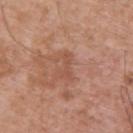biopsy_status: not biopsied; imaged during a skin examination
image:
  source: total-body photography crop
  field_of_view_mm: 15
site: upper back
lighting: white-light
lesion_size:
  long_diameter_mm_approx: 3.0
automated_metrics:
  vs_skin_darker_L: 6.0
  color_variation_0_10: 0.0
  nevus_likeness_0_100: 0
  lesion_detection_confidence_0_100: 100
patient:
  sex: male
  age_approx: 50